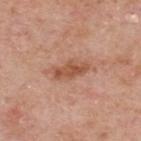<record>
  <lighting>white-light</lighting>
  <patient>
    <sex>male</sex>
    <age_approx>75</age_approx>
  </patient>
  <automated_metrics>
    <cielab_L>54</cielab_L>
    <cielab_a>23</cielab_a>
    <cielab_b>32</cielab_b>
    <vs_skin_darker_L>10.0</vs_skin_darker_L>
    <vs_skin_contrast_norm>7.5</vs_skin_contrast_norm>
    <nevus_likeness_0_100>0</nevus_likeness_0_100>
    <lesion_detection_confidence_0_100>100</lesion_detection_confidence_0_100>
  </automated_metrics>
  <lesion_size>
    <long_diameter_mm_approx>4.5</long_diameter_mm_approx>
  </lesion_size>
  <image>
    <source>total-body photography crop</source>
    <field_of_view_mm>15</field_of_view_mm>
  </image>
  <site>upper back</site>
</record>